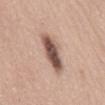Recorded during total-body skin imaging; not selected for excision or biopsy.
Imaged with white-light lighting.
A female subject about 50 years old.
Located on the mid back.
The lesion-visualizer software estimated a lesion area of about 11 mm², an eccentricity of roughly 0.9, and a symmetry-axis asymmetry near 0.25. The analysis additionally found a mean CIELAB color near L≈53 a*≈18 b*≈24, a lesion–skin lightness drop of about 18, and a normalized lesion–skin contrast near 11.5. It also reported a border-irregularity rating of about 3/10 and internal color variation of about 6 on a 0–10 scale.
A roughly 15 mm field-of-view crop from a total-body skin photograph.